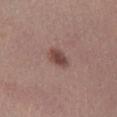<record>
<biopsy_status>not biopsied; imaged during a skin examination</biopsy_status>
<image>
  <source>total-body photography crop</source>
  <field_of_view_mm>15</field_of_view_mm>
</image>
<lesion_size>
  <long_diameter_mm_approx>3.0</long_diameter_mm_approx>
</lesion_size>
<site>right lower leg</site>
<patient>
  <sex>female</sex>
  <age_approx>25</age_approx>
</patient>
</record>